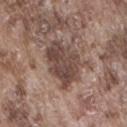biopsy_status: not biopsied; imaged during a skin examination
patient:
  sex: male
  age_approx: 75
lighting: white-light
image:
  source: total-body photography crop
  field_of_view_mm: 15
site: back
lesion_size:
  long_diameter_mm_approx: 5.5